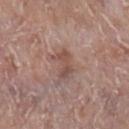| feature | finding |
|---|---|
| TBP lesion metrics | an area of roughly 5.5 mm², a shape eccentricity near 0.8, and a symmetry-axis asymmetry near 0.4; roughly 8 lightness units darker than nearby skin; border irregularity of about 5 on a 0–10 scale, a within-lesion color-variation index near 3/10, and peripheral color asymmetry of about 1; an automated nevus-likeness rating near 0 out of 100 and a lesion-detection confidence of about 100/100 |
| size | ~3 mm (longest diameter) |
| patient | male, about 45 years old |
| image source | ~15 mm crop, total-body skin-cancer survey |
| illumination | white-light illumination |
| body site | the right lower leg |